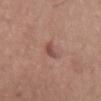Findings:
– biopsy status — no biopsy performed (imaged during a skin exam)
– diameter — about 2.5 mm
– patient — female, aged approximately 65
– image — total-body-photography crop, ~15 mm field of view
– image-analysis metrics — an eccentricity of roughly 0.9 and two-axis asymmetry of about 0.3; a mean CIELAB color near L≈49 a*≈24 b*≈25, about 9 CIELAB-L* units darker than the surrounding skin, and a normalized lesion–skin contrast near 7
– illumination — white-light
– location — the leg